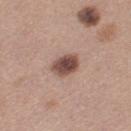– image · ~15 mm tile from a whole-body skin photo
– body site · the left thigh
– subject · female, roughly 30 years of age